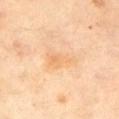Imaged during a routine full-body skin examination; the lesion was not biopsied and no histopathology is available. The tile uses cross-polarized illumination. Cropped from a total-body skin-imaging series; the visible field is about 15 mm. On the left upper arm. A male subject in their mid- to late 60s.